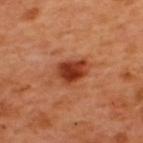Q: Lesion location?
A: the back
Q: How large is the lesion?
A: ≈4 mm
Q: Patient demographics?
A: male, aged approximately 50
Q: How was this image acquired?
A: ~15 mm crop, total-body skin-cancer survey
Q: How was the tile lit?
A: cross-polarized
Q: Automated lesion metrics?
A: a footprint of about 8 mm², a shape eccentricity near 0.7, and a symmetry-axis asymmetry near 0.25; a lesion color around L≈40 a*≈32 b*≈36 in CIELAB, roughly 14 lightness units darker than nearby skin, and a normalized lesion–skin contrast near 10.5; a color-variation rating of about 4.5/10 and peripheral color asymmetry of about 1.5